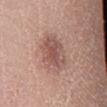Assessment:
This lesion was catalogued during total-body skin photography and was not selected for biopsy.
Image and clinical context:
A 15 mm close-up tile from a total-body photography series done for melanoma screening. Measured at roughly 5 mm in maximum diameter. A female subject aged 53–57. The lesion is located on the right lower leg.